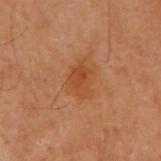Notes:
– biopsy status — no biopsy performed (imaged during a skin exam)
– body site — the right arm
– patient — female, aged 58–62
– illumination — cross-polarized
– image — ~15 mm tile from a whole-body skin photo
– lesion diameter — about 3.5 mm